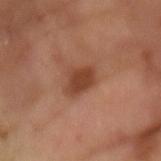Impression:
Imaged during a routine full-body skin examination; the lesion was not biopsied and no histopathology is available.